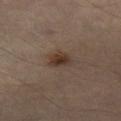{"automated_metrics": {"shape_asymmetry": 0.25, "nevus_likeness_0_100": 95}, "patient": {"sex": "male", "age_approx": 35}, "image": {"source": "total-body photography crop", "field_of_view_mm": 15}, "lighting": "cross-polarized", "lesion_size": {"long_diameter_mm_approx": 3.0}, "site": "right leg"}